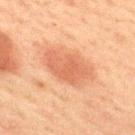The lesion was tiled from a total-body skin photograph and was not biopsied. About 5.5 mm across. A lesion tile, about 15 mm wide, cut from a 3D total-body photograph. This is a cross-polarized tile. A male subject approximately 60 years of age. Located on the upper back.